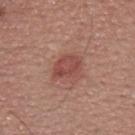Q: Was this lesion biopsied?
A: imaged on a skin check; not biopsied
Q: Lesion size?
A: ~3.5 mm (longest diameter)
Q: Automated lesion metrics?
A: a lesion area of about 8 mm², an outline eccentricity of about 0.7 (0 = round, 1 = elongated), and a symmetry-axis asymmetry near 0.25; an average lesion color of about L≈48 a*≈26 b*≈25 (CIELAB), about 9 CIELAB-L* units darker than the surrounding skin, and a normalized border contrast of about 6.5
Q: What lighting was used for the tile?
A: white-light
Q: How was this image acquired?
A: ~15 mm tile from a whole-body skin photo
Q: Lesion location?
A: the upper back
Q: Who is the patient?
A: male, in their 40s